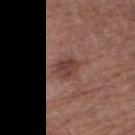<lesion>
<biopsy_status>not biopsied; imaged during a skin examination</biopsy_status>
</lesion>